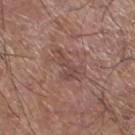<lesion>
  <lesion_size>
    <long_diameter_mm_approx>4.0</long_diameter_mm_approx>
  </lesion_size>
  <patient>
    <sex>male</sex>
    <age_approx>60</age_approx>
  </patient>
  <automated_metrics>
    <border_irregularity_0_10>7.5</border_irregularity_0_10>
    <color_variation_0_10>1.0</color_variation_0_10>
    <peripheral_color_asymmetry>0.5</peripheral_color_asymmetry>
  </automated_metrics>
  <image>
    <source>total-body photography crop</source>
    <field_of_view_mm>15</field_of_view_mm>
  </image>
  <lighting>white-light</lighting>
  <site>left lower leg</site>
</lesion>